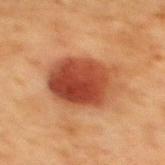{"biopsy_status": "not biopsied; imaged during a skin examination", "lighting": "cross-polarized", "image": {"source": "total-body photography crop", "field_of_view_mm": 15}, "site": "mid back", "patient": {"sex": "female", "age_approx": 40}}